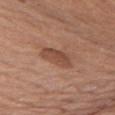Recorded during total-body skin imaging; not selected for excision or biopsy.
From the left upper arm.
A 15 mm close-up extracted from a 3D total-body photography capture.
Approximately 4 mm at its widest.
Captured under white-light illumination.
A female patient aged 63–67.
The lesion-visualizer software estimated peripheral color asymmetry of about 1.